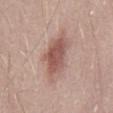Captured during whole-body skin photography for melanoma surveillance; the lesion was not biopsied.